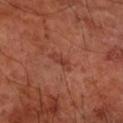Imaged during a routine full-body skin examination; the lesion was not biopsied and no histopathology is available. The total-body-photography lesion software estimated an outline eccentricity of about 0.9 (0 = round, 1 = elongated) and two-axis asymmetry of about 0.4. And it measured a lesion color around L≈38 a*≈26 b*≈29 in CIELAB, a lesion–skin lightness drop of about 7, and a normalized border contrast of about 5.5. The software also gave a nevus-likeness score of about 0/100 and lesion-presence confidence of about 100/100. From the right forearm. Captured under cross-polarized illumination. A male patient, in their 60s. A close-up tile cropped from a whole-body skin photograph, about 15 mm across.